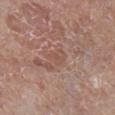Q: Was a biopsy performed?
A: imaged on a skin check; not biopsied
Q: How was the tile lit?
A: white-light
Q: How was this image acquired?
A: 15 mm crop, total-body photography
Q: What is the lesion's diameter?
A: ~3 mm (longest diameter)
Q: Who is the patient?
A: male, approximately 85 years of age or older
Q: What is the anatomic site?
A: the right lower leg